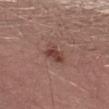Automated image analysis of the tile measured a lesion area of about 5 mm², a shape eccentricity near 0.8, and two-axis asymmetry of about 0.25. And it measured an average lesion color of about L≈42 a*≈22 b*≈23 (CIELAB) and a lesion-to-skin contrast of about 7.5 (normalized; higher = more distinct). The software also gave a detector confidence of about 100 out of 100 that the crop contains a lesion.
Approximately 3 mm at its widest.
A close-up tile cropped from a whole-body skin photograph, about 15 mm across.
The subject is a male in their 50s.
The lesion is located on the left thigh.
The tile uses white-light illumination.